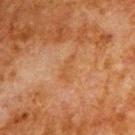<tbp_lesion>
  <biopsy_status>not biopsied; imaged during a skin examination</biopsy_status>
  <site>upper back</site>
  <image>
    <source>total-body photography crop</source>
    <field_of_view_mm>15</field_of_view_mm>
  </image>
  <patient>
    <sex>male</sex>
    <age_approx>80</age_approx>
  </patient>
  <lighting>cross-polarized</lighting>
</tbp_lesion>